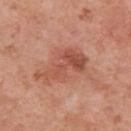<lesion>
<biopsy_status>not biopsied; imaged during a skin examination</biopsy_status>
<patient>
  <sex>female</sex>
  <age_approx>40</age_approx>
</patient>
<site>arm</site>
<automated_metrics>
  <eccentricity>0.85</eccentricity>
  <shape_asymmetry>0.55</shape_asymmetry>
  <color_variation_0_10>5.0</color_variation_0_10>
  <peripheral_color_asymmetry>2.0</peripheral_color_asymmetry>
</automated_metrics>
<image>
  <source>total-body photography crop</source>
  <field_of_view_mm>15</field_of_view_mm>
</image>
<lighting>white-light</lighting>
</lesion>